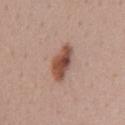{"biopsy_status": "not biopsied; imaged during a skin examination", "patient": {"sex": "female", "age_approx": 45}, "lesion_size": {"long_diameter_mm_approx": 4.5}, "automated_metrics": {"area_mm2_approx": 8.5, "shape_asymmetry": 0.2, "cielab_L": 50, "cielab_a": 21, "cielab_b": 28, "vs_skin_darker_L": 14.0, "vs_skin_contrast_norm": 10.0, "border_irregularity_0_10": 2.5, "peripheral_color_asymmetry": 1.5, "nevus_likeness_0_100": 90, "lesion_detection_confidence_0_100": 100}, "site": "abdomen", "image": {"source": "total-body photography crop", "field_of_view_mm": 15}}